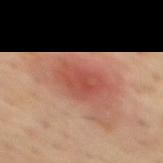Part of a total-body skin-imaging series; this lesion was reviewed on a skin check and was not flagged for biopsy. The tile uses cross-polarized illumination. Located on the back. A roughly 15 mm field-of-view crop from a total-body skin photograph. Approximately 4.5 mm at its widest. A male patient aged 53 to 57.